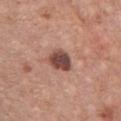| feature | finding |
|---|---|
| workup | total-body-photography surveillance lesion; no biopsy |
| TBP lesion metrics | a lesion area of about 7 mm², an outline eccentricity of about 0.65 (0 = round, 1 = elongated), and two-axis asymmetry of about 0.2; a border-irregularity rating of about 2/10, a within-lesion color-variation index near 3.5/10, and radial color variation of about 1; an automated nevus-likeness rating near 75 out of 100 and a lesion-detection confidence of about 100/100 |
| site | the chest |
| imaging modality | 15 mm crop, total-body photography |
| patient | male, in their 60s |
| diameter | about 3.5 mm |